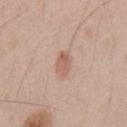notes: imaged on a skin check; not biopsied | patient: male, about 50 years old | location: the front of the torso | lighting: white-light | image-analysis metrics: an outline eccentricity of about 0.8 (0 = round, 1 = elongated) and a symmetry-axis asymmetry near 0.25; an average lesion color of about L≈59 a*≈22 b*≈27 (CIELAB) and a normalized border contrast of about 6.5 | lesion size: about 2.5 mm | image source: ~15 mm tile from a whole-body skin photo.